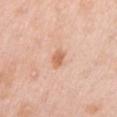| feature | finding |
|---|---|
| workup | catalogued during a skin exam; not biopsied |
| image source | 15 mm crop, total-body photography |
| subject | female, roughly 50 years of age |
| anatomic site | the right upper arm |